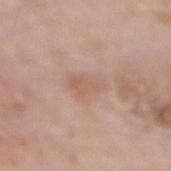Recorded during total-body skin imaging; not selected for excision or biopsy. The lesion is on the upper back. The lesion's longest dimension is about 3.5 mm. A female subject in their 50s. A region of skin cropped from a whole-body photographic capture, roughly 15 mm wide. The lesion-visualizer software estimated a footprint of about 6 mm² and a symmetry-axis asymmetry near 0.3. The analysis additionally found a lesion-detection confidence of about 100/100.